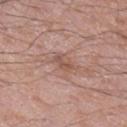biopsy_status: not biopsied; imaged during a skin examination
lighting: white-light
site: right lower leg
patient:
  sex: male
  age_approx: 60
image:
  source: total-body photography crop
  field_of_view_mm: 15